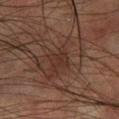- follow-up — imaged on a skin check; not biopsied
- patient — male, approximately 75 years of age
- imaging modality — 15 mm crop, total-body photography
- location — the left forearm